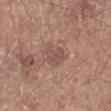{"biopsy_status": "not biopsied; imaged during a skin examination", "site": "left lower leg", "automated_metrics": {"cielab_L": 50, "cielab_a": 21, "cielab_b": 23, "vs_skin_darker_L": 7.0, "vs_skin_contrast_norm": 5.5, "border_irregularity_0_10": 3.5, "color_variation_0_10": 3.0, "peripheral_color_asymmetry": 1.0, "nevus_likeness_0_100": 0, "lesion_detection_confidence_0_100": 100}, "image": {"source": "total-body photography crop", "field_of_view_mm": 15}, "patient": {"sex": "male", "age_approx": 75}}